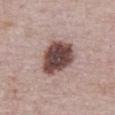Q: Was a biopsy performed?
A: catalogued during a skin exam; not biopsied
Q: Lesion size?
A: about 5 mm
Q: Lesion location?
A: the abdomen
Q: What are the patient's age and sex?
A: male, in their mid-70s
Q: Automated lesion metrics?
A: an area of roughly 15 mm², an outline eccentricity of about 0.6 (0 = round, 1 = elongated), and a shape-asymmetry score of about 0.2 (0 = symmetric); roughly 20 lightness units darker than nearby skin and a normalized lesion–skin contrast near 14
Q: Illumination type?
A: white-light
Q: How was this image acquired?
A: ~15 mm crop, total-body skin-cancer survey